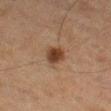The lesion was tiled from a total-body skin photograph and was not biopsied. The lesion is on the left thigh. A 15 mm close-up extracted from a 3D total-body photography capture. The tile uses cross-polarized illumination. A male patient, roughly 75 years of age. An algorithmic analysis of the crop reported internal color variation of about 3 on a 0–10 scale. And it measured an automated nevus-likeness rating near 100 out of 100 and a detector confidence of about 100 out of 100 that the crop contains a lesion. Measured at roughly 2.5 mm in maximum diameter.